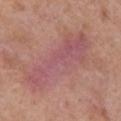Image and clinical context:
Measured at roughly 10 mm in maximum diameter. A 15 mm crop from a total-body photograph taken for skin-cancer surveillance. The subject is a female aged approximately 40. Automated image analysis of the tile measured an area of roughly 31 mm² and an eccentricity of roughly 0.95. The analysis additionally found a normalized lesion–skin contrast near 6. The lesion is located on the right upper arm.